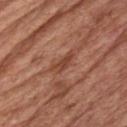Findings:
• workup · total-body-photography surveillance lesion; no biopsy
• TBP lesion metrics · a shape-asymmetry score of about 0.5 (0 = symmetric); an average lesion color of about L≈44 a*≈25 b*≈31 (CIELAB), about 9 CIELAB-L* units darker than the surrounding skin, and a normalized border contrast of about 7
• lighting · white-light illumination
• subject · male, about 60 years old
• lesion diameter · ≈3 mm
• imaging modality · total-body-photography crop, ~15 mm field of view
• anatomic site · the chest